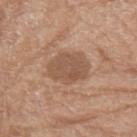| field | value |
|---|---|
| follow-up | catalogued during a skin exam; not biopsied |